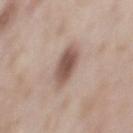Imaged during a routine full-body skin examination; the lesion was not biopsied and no histopathology is available.
A 15 mm close-up tile from a total-body photography series done for melanoma screening.
The subject is a male about 55 years old.
The lesion is located on the mid back.